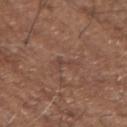biopsy status: catalogued during a skin exam; not biopsied
location: the arm
subject: male, in their mid-70s
illumination: white-light
lesion diameter: ~2.5 mm (longest diameter)
imaging modality: ~15 mm tile from a whole-body skin photo